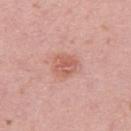{
  "biopsy_status": "not biopsied; imaged during a skin examination",
  "site": "right upper arm",
  "lighting": "white-light",
  "automated_metrics": {
    "area_mm2_approx": 7.0,
    "eccentricity": 0.45,
    "shape_asymmetry": 0.15,
    "lesion_detection_confidence_0_100": 100
  },
  "image": {
    "source": "total-body photography crop",
    "field_of_view_mm": 15
  },
  "patient": {
    "sex": "female",
    "age_approx": 30
  },
  "lesion_size": {
    "long_diameter_mm_approx": 3.0
  }
}